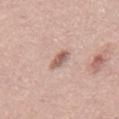Impression: Imaged during a routine full-body skin examination; the lesion was not biopsied and no histopathology is available. Background: On the mid back. The recorded lesion diameter is about 2.5 mm. A roughly 15 mm field-of-view crop from a total-body skin photograph. The tile uses white-light illumination. The total-body-photography lesion software estimated a lesion area of about 4 mm², a shape eccentricity near 0.75, and a shape-asymmetry score of about 0.15 (0 = symmetric). It also reported a mean CIELAB color near L≈59 a*≈19 b*≈25, roughly 12 lightness units darker than nearby skin, and a normalized border contrast of about 7.5. It also reported a border-irregularity rating of about 1.5/10, a color-variation rating of about 3.5/10, and a peripheral color-asymmetry measure near 1. The analysis additionally found a nevus-likeness score of about 65/100 and a lesion-detection confidence of about 100/100. A male patient, in their mid-40s.